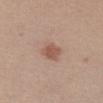biopsy status: imaged on a skin check; not biopsied
location: the chest
automated metrics: a mean CIELAB color near L≈54 a*≈21 b*≈28, roughly 10 lightness units darker than nearby skin, and a normalized border contrast of about 7
image source: 15 mm crop, total-body photography
patient: female, in their mid-30s
illumination: white-light illumination
lesion size: about 2.5 mm